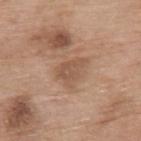• workup — imaged on a skin check; not biopsied
• subject — female, aged 73–77
• image — ~15 mm crop, total-body skin-cancer survey
• illumination — white-light illumination
• location — the upper back
• automated lesion analysis — an area of roughly 6 mm², an outline eccentricity of about 0.75 (0 = round, 1 = elongated), and a symmetry-axis asymmetry near 0.35; a lesion color around L≈53 a*≈19 b*≈30 in CIELAB, roughly 8 lightness units darker than nearby skin, and a lesion-to-skin contrast of about 5.5 (normalized; higher = more distinct); a nevus-likeness score of about 5/100 and a lesion-detection confidence of about 100/100